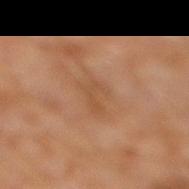follow-up = catalogued during a skin exam; not biopsied | image-analysis metrics = an area of roughly 6 mm² and two-axis asymmetry of about 0.4; an average lesion color of about L≈48 a*≈19 b*≈32 (CIELAB) and about 5 CIELAB-L* units darker than the surrounding skin; a border-irregularity index near 5/10 and internal color variation of about 2 on a 0–10 scale | acquisition = 15 mm crop, total-body photography | site = the leg | illumination = cross-polarized | patient = male, in their 60s.